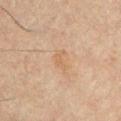Q: Was this lesion biopsied?
A: imaged on a skin check; not biopsied
Q: How was this image acquired?
A: ~15 mm crop, total-body skin-cancer survey
Q: Lesion location?
A: the chest
Q: What lighting was used for the tile?
A: cross-polarized illumination
Q: Who is the patient?
A: male, roughly 60 years of age
Q: How large is the lesion?
A: ~2.5 mm (longest diameter)
Q: Automated lesion metrics?
A: a mean CIELAB color near L≈51 a*≈16 b*≈30, about 5 CIELAB-L* units darker than the surrounding skin, and a normalized border contrast of about 4.5; an automated nevus-likeness rating near 0 out of 100 and lesion-presence confidence of about 100/100